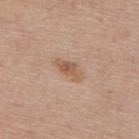| feature | finding |
|---|---|
| notes | imaged on a skin check; not biopsied |
| lighting | white-light |
| image-analysis metrics | a footprint of about 5 mm², an eccentricity of roughly 0.9, and a shape-asymmetry score of about 0.3 (0 = symmetric); a color-variation rating of about 3.5/10 and radial color variation of about 1; a classifier nevus-likeness of about 40/100 and lesion-presence confidence of about 100/100 |
| anatomic site | the upper back |
| image source | ~15 mm crop, total-body skin-cancer survey |
| lesion size | about 3.5 mm |
| subject | female, aged around 50 |